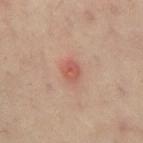No biopsy was performed on this lesion — it was imaged during a full skin examination and was not determined to be concerning.
The total-body-photography lesion software estimated a lesion area of about 4 mm² and a shape-asymmetry score of about 0.2 (0 = symmetric).
Located on the mid back.
The patient is a male aged 58 to 62.
This image is a 15 mm lesion crop taken from a total-body photograph.
Measured at roughly 2.5 mm in maximum diameter.